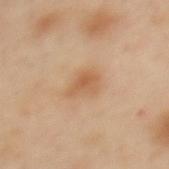Part of a total-body skin-imaging series; this lesion was reviewed on a skin check and was not flagged for biopsy. From the upper back. A region of skin cropped from a whole-body photographic capture, roughly 15 mm wide. A female patient, aged 38 to 42.